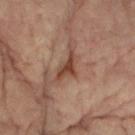Impression: Part of a total-body skin-imaging series; this lesion was reviewed on a skin check and was not flagged for biopsy. Background: A female subject, aged approximately 80. From the arm. This image is a 15 mm lesion crop taken from a total-body photograph.